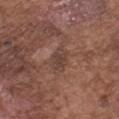Measured at roughly 3 mm in maximum diameter.
From the chest.
A region of skin cropped from a whole-body photographic capture, roughly 15 mm wide.
The subject is a male in their mid-70s.
The total-body-photography lesion software estimated a lesion area of about 5 mm² and a shape-asymmetry score of about 0.2 (0 = symmetric). The software also gave an automated nevus-likeness rating near 0 out of 100 and lesion-presence confidence of about 90/100.